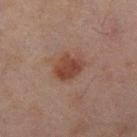Case summary:
• notes — imaged on a skin check; not biopsied
• acquisition — ~15 mm tile from a whole-body skin photo
• patient — male, in their mid-40s
• body site — the right thigh
• size — ~3.5 mm (longest diameter)
• tile lighting — cross-polarized illumination